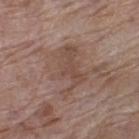This lesion was catalogued during total-body skin photography and was not selected for biopsy. This image is a 15 mm lesion crop taken from a total-body photograph. Approximately 5.5 mm at its widest. The lesion is on the left thigh. The subject is a female about 70 years old. The tile uses white-light illumination.